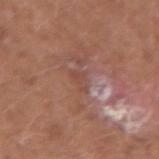workup — imaged on a skin check; not biopsied | lesion diameter — about 2.5 mm | anatomic site — the left forearm | acquisition — 15 mm crop, total-body photography | patient — male, roughly 60 years of age | tile lighting — white-light.